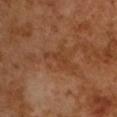The lesion was tiled from a total-body skin photograph and was not biopsied.
A 15 mm close-up extracted from a 3D total-body photography capture.
The total-body-photography lesion software estimated an average lesion color of about L≈38 a*≈22 b*≈33 (CIELAB) and about 6 CIELAB-L* units darker than the surrounding skin. The analysis additionally found an automated nevus-likeness rating near 0 out of 100.
Captured under cross-polarized illumination.
Approximately 3.5 mm at its widest.
The subject is a male about 65 years old.